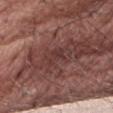Findings:
– notes · total-body-photography surveillance lesion; no biopsy
– acquisition · 15 mm crop, total-body photography
– subject · male, in their mid- to late 70s
– site · the right thigh